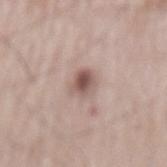Notes:
- notes — catalogued during a skin exam; not biopsied
- lesion diameter — ≈2.5 mm
- anatomic site — the mid back
- acquisition — ~15 mm crop, total-body skin-cancer survey
- patient — male, approximately 65 years of age